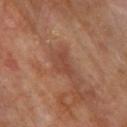Assessment: Imaged during a routine full-body skin examination; the lesion was not biopsied and no histopathology is available. Context: A close-up tile cropped from a whole-body skin photograph, about 15 mm across. A male patient, approximately 70 years of age. The lesion-visualizer software estimated an average lesion color of about L≈43 a*≈22 b*≈29 (CIELAB), a lesion–skin lightness drop of about 7, and a lesion-to-skin contrast of about 5.5 (normalized; higher = more distinct). The analysis additionally found a color-variation rating of about 2/10. The analysis additionally found a nevus-likeness score of about 0/100 and a lesion-detection confidence of about 100/100. The lesion is located on the upper back. Captured under cross-polarized illumination. Measured at roughly 3.5 mm in maximum diameter.